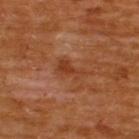Impression: Recorded during total-body skin imaging; not selected for excision or biopsy. Image and clinical context: A 15 mm crop from a total-body photograph taken for skin-cancer surveillance. A male subject, aged 63–67. The lesion-visualizer software estimated an eccentricity of roughly 0.85 and a symmetry-axis asymmetry near 0.5. The software also gave a lesion color around L≈38 a*≈26 b*≈34 in CIELAB and about 7 CIELAB-L* units darker than the surrounding skin. It also reported a lesion-detection confidence of about 100/100. Located on the upper back.